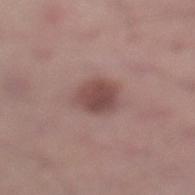follow-up: imaged on a skin check; not biopsied
TBP lesion metrics: a footprint of about 8 mm² and an eccentricity of roughly 0.65; a mean CIELAB color near L≈47 a*≈20 b*≈21 and roughly 11 lightness units darker than nearby skin; a nevus-likeness score of about 85/100 and a detector confidence of about 100 out of 100 that the crop contains a lesion
location: the left lower leg
imaging modality: 15 mm crop, total-body photography
lesion diameter: ≈3.5 mm
tile lighting: white-light
patient: male, aged 33 to 37